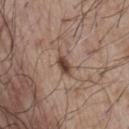Notes:
- notes — no biopsy performed (imaged during a skin exam)
- diameter — about 3 mm
- patient — male, in their mid-70s
- imaging modality — 15 mm crop, total-body photography
- tile lighting — white-light
- location — the chest